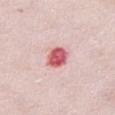Q: Was a biopsy performed?
A: total-body-photography surveillance lesion; no biopsy
Q: Where on the body is the lesion?
A: the chest
Q: Who is the patient?
A: female, approximately 65 years of age
Q: Lesion size?
A: ~3 mm (longest diameter)
Q: Illumination type?
A: white-light illumination
Q: What kind of image is this?
A: ~15 mm tile from a whole-body skin photo